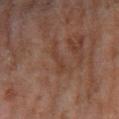Case summary:
• workup: catalogued during a skin exam; not biopsied